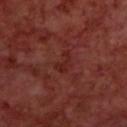size=about 2.5 mm | anatomic site=the upper back | lighting=cross-polarized illumination | image source=~15 mm tile from a whole-body skin photo | patient=male, in their 70s.